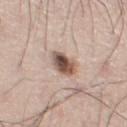Notes:
- biopsy status — catalogued during a skin exam; not biopsied
- imaging modality — ~15 mm tile from a whole-body skin photo
- patient — male, aged approximately 70
- tile lighting — white-light
- anatomic site — the right leg
- lesion diameter — ≈3 mm